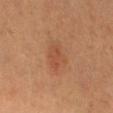notes=imaged on a skin check; not biopsied
tile lighting=cross-polarized
patient=female, aged 28 to 32
image=total-body-photography crop, ~15 mm field of view
anatomic site=the leg
diameter=~3.5 mm (longest diameter)